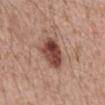{"biopsy_status": "not biopsied; imaged during a skin examination", "patient": {"sex": "male", "age_approx": 60}, "automated_metrics": {"area_mm2_approx": 12.0, "eccentricity": 0.65, "shape_asymmetry": 0.2, "cielab_L": 46, "cielab_a": 22, "cielab_b": 26, "vs_skin_contrast_norm": 10.5, "nevus_likeness_0_100": 90, "lesion_detection_confidence_0_100": 100}, "lesion_size": {"long_diameter_mm_approx": 4.5}, "image": {"source": "total-body photography crop", "field_of_view_mm": 15}, "site": "abdomen"}